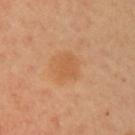Clinical impression: The lesion was tiled from a total-body skin photograph and was not biopsied. Acquisition and patient details: Captured under cross-polarized illumination. Located on the left upper arm. Cropped from a total-body skin-imaging series; the visible field is about 15 mm. An algorithmic analysis of the crop reported a mean CIELAB color near L≈59 a*≈24 b*≈40, a lesion–skin lightness drop of about 6, and a normalized border contrast of about 5. The software also gave border irregularity of about 2.5 on a 0–10 scale, a within-lesion color-variation index near 1.5/10, and peripheral color asymmetry of about 0.5. A female patient aged approximately 60. About 3 mm across.